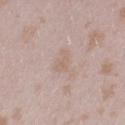Impression:
Recorded during total-body skin imaging; not selected for excision or biopsy.
Image and clinical context:
A female subject in their mid-20s. A 15 mm close-up extracted from a 3D total-body photography capture. This is a white-light tile. On the left thigh. Automated tile analysis of the lesion measured a footprint of about 3.5 mm², a shape eccentricity near 0.8, and a symmetry-axis asymmetry near 0.45. It also reported a classifier nevus-likeness of about 0/100 and a lesion-detection confidence of about 100/100.